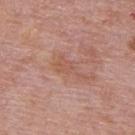Clinical summary:
Automated tile analysis of the lesion measured a lesion area of about 4.5 mm², a shape eccentricity near 0.9, and two-axis asymmetry of about 0.55. Cropped from a total-body skin-imaging series; the visible field is about 15 mm. A male subject, in their mid-70s. Measured at roughly 4 mm in maximum diameter. The tile uses white-light illumination. The lesion is on the upper back.